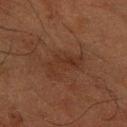This lesion was catalogued during total-body skin photography and was not selected for biopsy. A male patient approximately 60 years of age. The tile uses cross-polarized illumination. Approximately 4.5 mm at its widest. The lesion is on the right forearm. A 15 mm close-up extracted from a 3D total-body photography capture.